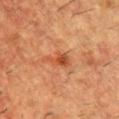workup=no biopsy performed (imaged during a skin exam) | subject=male, approximately 55 years of age | anatomic site=the upper back | image source=15 mm crop, total-body photography.